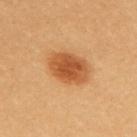Findings:
– workup · imaged on a skin check; not biopsied
– TBP lesion metrics · an eccentricity of roughly 0.7; a mean CIELAB color near L≈53 a*≈26 b*≈42 and a normalized border contrast of about 9; a border-irregularity rating of about 1/10, a within-lesion color-variation index near 3.5/10, and peripheral color asymmetry of about 1; a classifier nevus-likeness of about 100/100 and a detector confidence of about 100 out of 100 that the crop contains a lesion
– imaging modality · ~15 mm crop, total-body skin-cancer survey
– illumination · cross-polarized
– subject · female, about 25 years old
– body site · the back
– lesion size · ~4.5 mm (longest diameter)